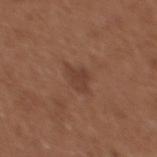Part of a total-body skin-imaging series; this lesion was reviewed on a skin check and was not flagged for biopsy. A female subject, aged around 35. A region of skin cropped from a whole-body photographic capture, roughly 15 mm wide. On the chest.